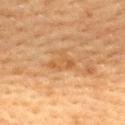Recorded during total-body skin imaging; not selected for excision or biopsy. A female subject, roughly 55 years of age. A lesion tile, about 15 mm wide, cut from a 3D total-body photograph. On the upper back. About 3.5 mm across.